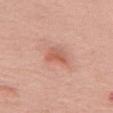| key | value |
|---|---|
| biopsy status | catalogued during a skin exam; not biopsied |
| automated lesion analysis | a lesion area of about 4 mm², an eccentricity of roughly 0.6, and a shape-asymmetry score of about 0.4 (0 = symmetric); an average lesion color of about L≈58 a*≈28 b*≈31 (CIELAB), about 9 CIELAB-L* units darker than the surrounding skin, and a lesion-to-skin contrast of about 6.5 (normalized; higher = more distinct); a nevus-likeness score of about 65/100 |
| lesion diameter | ~3 mm (longest diameter) |
| site | the front of the torso |
| patient | female, about 65 years old |
| tile lighting | white-light |
| acquisition | ~15 mm crop, total-body skin-cancer survey |